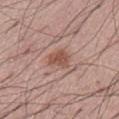Case summary:
- notes: total-body-photography surveillance lesion; no biopsy
- image-analysis metrics: a detector confidence of about 100 out of 100 that the crop contains a lesion
- site: the abdomen
- tile lighting: white-light
- image source: ~15 mm crop, total-body skin-cancer survey
- lesion diameter: about 2.5 mm
- subject: male, aged around 55